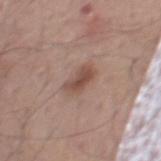This lesion was catalogued during total-body skin photography and was not selected for biopsy. The patient is a male aged 53 to 57. On the chest. Automated image analysis of the tile measured an area of roughly 6 mm², an outline eccentricity of about 0.85 (0 = round, 1 = elongated), and a shape-asymmetry score of about 0.25 (0 = symmetric). And it measured a mean CIELAB color near L≈50 a*≈19 b*≈25 and a normalized border contrast of about 7.5. It also reported a nevus-likeness score of about 60/100 and a detector confidence of about 100 out of 100 that the crop contains a lesion. A close-up tile cropped from a whole-body skin photograph, about 15 mm across. This is a white-light tile.